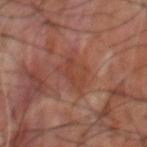<lesion>
<biopsy_status>not biopsied; imaged during a skin examination</biopsy_status>
<lighting>cross-polarized</lighting>
<image>
  <source>total-body photography crop</source>
  <field_of_view_mm>15</field_of_view_mm>
</image>
<site>upper back</site>
<lesion_size>
  <long_diameter_mm_approx>4.5</long_diameter_mm_approx>
</lesion_size>
<patient>
  <sex>male</sex>
  <age_approx>60</age_approx>
</patient>
<automated_metrics>
  <area_mm2_approx>8.5</area_mm2_approx>
  <eccentricity>0.8</eccentricity>
  <cielab_L>38</cielab_L>
  <cielab_a>22</cielab_a>
  <cielab_b>25</cielab_b>
  <vs_skin_darker_L>6.0</vs_skin_darker_L>
  <lesion_detection_confidence_0_100>95</lesion_detection_confidence_0_100>
</automated_metrics>
</lesion>